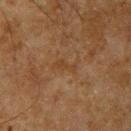workup: imaged on a skin check; not biopsied | subject: male, aged 63 to 67 | lesion diameter: ~3 mm (longest diameter) | anatomic site: the left upper arm | acquisition: total-body-photography crop, ~15 mm field of view | illumination: cross-polarized illumination.